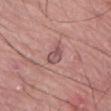This lesion was catalogued during total-body skin photography and was not selected for biopsy. The total-body-photography lesion software estimated an area of roughly 4 mm², an outline eccentricity of about 0.7 (0 = round, 1 = elongated), and a symmetry-axis asymmetry near 0.35. The analysis additionally found a border-irregularity index near 3.5/10, a within-lesion color-variation index near 3/10, and radial color variation of about 1. Located on the lower back. A male subject aged approximately 75. This is a white-light tile. The recorded lesion diameter is about 2.5 mm. A 15 mm close-up extracted from a 3D total-body photography capture.